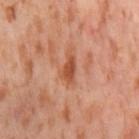Q: Was a biopsy performed?
A: catalogued during a skin exam; not biopsied
Q: Patient demographics?
A: female, aged 53 to 57
Q: What kind of image is this?
A: 15 mm crop, total-body photography
Q: What is the lesion's diameter?
A: ≈3 mm
Q: Lesion location?
A: the leg